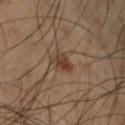Captured during whole-body skin photography for melanoma surveillance; the lesion was not biopsied. Cropped from a total-body skin-imaging series; the visible field is about 15 mm. The lesion's longest dimension is about 3 mm. The lesion is located on the left lower leg. Automated tile analysis of the lesion measured border irregularity of about 2.5 on a 0–10 scale, a within-lesion color-variation index near 2/10, and radial color variation of about 0.5. It also reported a detector confidence of about 100 out of 100 that the crop contains a lesion. A male patient roughly 50 years of age. The tile uses cross-polarized illumination.